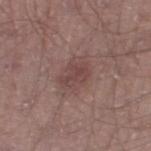Acquisition and patient details:
Measured at roughly 3 mm in maximum diameter. This is a white-light tile. A 15 mm close-up extracted from a 3D total-body photography capture. On the right thigh. The patient is a male roughly 55 years of age.